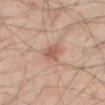Recorded during total-body skin imaging; not selected for excision or biopsy.
A male subject aged 63 to 67.
A region of skin cropped from a whole-body photographic capture, roughly 15 mm wide.
The lesion is on the right thigh.